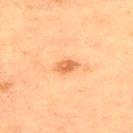<record>
<biopsy_status>not biopsied; imaged during a skin examination</biopsy_status>
<lesion_size>
  <long_diameter_mm_approx>3.0</long_diameter_mm_approx>
</lesion_size>
<image>
  <source>total-body photography crop</source>
  <field_of_view_mm>15</field_of_view_mm>
</image>
<lighting>cross-polarized</lighting>
<patient>
  <sex>male</sex>
  <age_approx>50</age_approx>
</patient>
<site>back</site>
<automated_metrics>
  <cielab_L>52</cielab_L>
  <cielab_a>23</cielab_a>
  <cielab_b>37</cielab_b>
  <vs_skin_contrast_norm>7.5</vs_skin_contrast_norm>
  <color_variation_0_10>3.0</color_variation_0_10>
  <nevus_likeness_0_100>85</nevus_likeness_0_100>
  <lesion_detection_confidence_0_100>100</lesion_detection_confidence_0_100>
</automated_metrics>
</record>